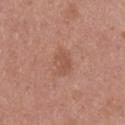Imaged during a routine full-body skin examination; the lesion was not biopsied and no histopathology is available. A roughly 15 mm field-of-view crop from a total-body skin photograph. The total-body-photography lesion software estimated a mean CIELAB color near L≈53 a*≈23 b*≈29 and a lesion-to-skin contrast of about 5 (normalized; higher = more distinct). Longest diameter approximately 3.5 mm. A male patient, in their mid-20s. From the upper back.